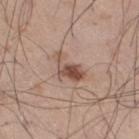<lesion>
<biopsy_status>not biopsied; imaged during a skin examination</biopsy_status>
<lesion_size>
  <long_diameter_mm_approx>4.5</long_diameter_mm_approx>
</lesion_size>
<site>right thigh</site>
<lighting>white-light</lighting>
<image>
  <source>total-body photography crop</source>
  <field_of_view_mm>15</field_of_view_mm>
</image>
<patient>
  <sex>male</sex>
  <age_approx>45</age_approx>
</patient>
</lesion>